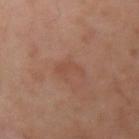notes: no biopsy performed (imaged during a skin exam) | lighting: cross-polarized | lesion size: ≈3 mm | image source: total-body-photography crop, ~15 mm field of view | subject: male, aged around 50 | TBP lesion metrics: a footprint of about 3.5 mm²; a lesion color around L≈47 a*≈22 b*≈28 in CIELAB and roughly 5 lightness units darker than nearby skin; border irregularity of about 6.5 on a 0–10 scale and a color-variation rating of about 0/10; an automated nevus-likeness rating near 0 out of 100 and lesion-presence confidence of about 100/100 | location: the arm.